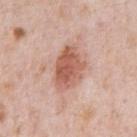biopsy_status: not biopsied; imaged during a skin examination
lighting: white-light
image:
  source: total-body photography crop
  field_of_view_mm: 15
lesion_size:
  long_diameter_mm_approx: 5.5
patient:
  sex: male
  age_approx: 80
site: chest